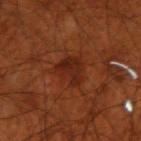image = 15 mm crop, total-body photography; location = the right upper arm; TBP lesion metrics = a nevus-likeness score of about 95/100 and a lesion-detection confidence of about 100/100; patient = male, aged 68 to 72; lesion size = ~3.5 mm (longest diameter); illumination = cross-polarized illumination.